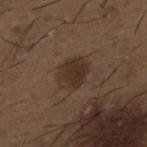| field | value |
|---|---|
| notes | no biopsy performed (imaged during a skin exam) |
| acquisition | ~15 mm crop, total-body skin-cancer survey |
| anatomic site | the upper back |
| lighting | white-light illumination |
| lesion size | ≈4 mm |
| subject | male, about 50 years old |